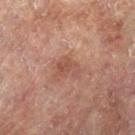workup: catalogued during a skin exam; not biopsied
lesion diameter: ~3 mm (longest diameter)
image: ~15 mm crop, total-body skin-cancer survey
TBP lesion metrics: an area of roughly 5 mm² and a shape eccentricity near 0.75
subject: female, about 80 years old
illumination: cross-polarized
anatomic site: the left leg